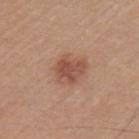The lesion was photographed on a routine skin check and not biopsied; there is no pathology result.
The total-body-photography lesion software estimated a footprint of about 8.5 mm² and two-axis asymmetry of about 0.25. It also reported a border-irregularity index near 3/10 and a color-variation rating of about 3/10. It also reported a nevus-likeness score of about 90/100 and lesion-presence confidence of about 100/100.
Imaged with white-light lighting.
This image is a 15 mm lesion crop taken from a total-body photograph.
The subject is a male aged 38 to 42.
Located on the arm.
Measured at roughly 4 mm in maximum diameter.